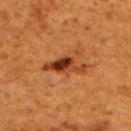biopsy status = no biopsy performed (imaged during a skin exam)
illumination = cross-polarized illumination
anatomic site = the upper back
image-analysis metrics = a lesion area of about 7.5 mm², an eccentricity of roughly 0.9, and a symmetry-axis asymmetry near 0.4
patient = female, aged 48 to 52
acquisition = 15 mm crop, total-body photography